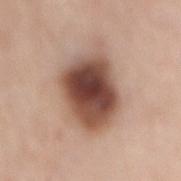Assessment: The lesion was tiled from a total-body skin photograph and was not biopsied. Clinical summary: From the mid back. A male subject aged 78 to 82. This image is a 15 mm lesion crop taken from a total-body photograph. Approximately 8 mm at its widest. The lesion-visualizer software estimated a footprint of about 29 mm², an outline eccentricity of about 0.7 (0 = round, 1 = elongated), and a symmetry-axis asymmetry near 0.2. The software also gave a lesion color around L≈49 a*≈20 b*≈26 in CIELAB and about 19 CIELAB-L* units darker than the surrounding skin. Imaged with white-light lighting.